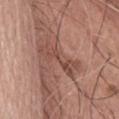Clinical impression:
Part of a total-body skin-imaging series; this lesion was reviewed on a skin check and was not flagged for biopsy.
Clinical summary:
The patient is a female roughly 75 years of age. Cropped from a total-body skin-imaging series; the visible field is about 15 mm. The lesion is on the abdomen. Approximately 5.5 mm at its widest.